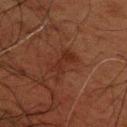workup — total-body-photography surveillance lesion; no biopsy
imaging modality — 15 mm crop, total-body photography
location — the upper back
subject — male, aged around 60
illumination — cross-polarized illumination
diameter — ≈4 mm
automated lesion analysis — an average lesion color of about L≈22 a*≈19 b*≈23 (CIELAB), roughly 5 lightness units darker than nearby skin, and a normalized border contrast of about 6; border irregularity of about 6 on a 0–10 scale, a color-variation rating of about 1.5/10, and a peripheral color-asymmetry measure near 0.5